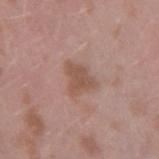follow-up: catalogued during a skin exam; not biopsied | patient: male, approximately 40 years of age | site: the right upper arm | lesion size: ~3.5 mm (longest diameter) | tile lighting: white-light illumination | image-analysis metrics: a lesion area of about 8.5 mm² and an outline eccentricity of about 0.6 (0 = round, 1 = elongated); a border-irregularity index near 3.5/10 | imaging modality: ~15 mm tile from a whole-body skin photo.